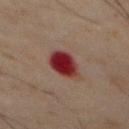workup: total-body-photography surveillance lesion; no biopsy | image source: 15 mm crop, total-body photography | lesion size: about 4 mm | tile lighting: cross-polarized | patient: male, about 70 years old | body site: the back.